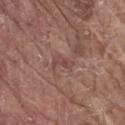The lesion's longest dimension is about 3 mm. Located on the leg. The subject is a male aged 78–82. Cropped from a whole-body photographic skin survey; the tile spans about 15 mm.